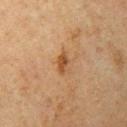{
  "image": {
    "source": "total-body photography crop",
    "field_of_view_mm": 15
  },
  "lesion_size": {
    "long_diameter_mm_approx": 3.0
  },
  "lighting": "cross-polarized",
  "site": "left upper arm",
  "patient": {
    "sex": "female",
    "age_approx": 60
  }
}